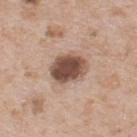Part of a total-body skin-imaging series; this lesion was reviewed on a skin check and was not flagged for biopsy.
The total-body-photography lesion software estimated an area of roughly 12 mm² and two-axis asymmetry of about 0.15. The analysis additionally found internal color variation of about 5 on a 0–10 scale and peripheral color asymmetry of about 1.5. The analysis additionally found an automated nevus-likeness rating near 80 out of 100 and a lesion-detection confidence of about 100/100.
Captured under white-light illumination.
Located on the upper back.
The subject is a male aged approximately 65.
A 15 mm close-up extracted from a 3D total-body photography capture.